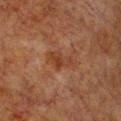– notes — catalogued during a skin exam; not biopsied
– image source — ~15 mm crop, total-body skin-cancer survey
– site — the chest
– patient — female, aged approximately 55
– illumination — cross-polarized illumination
– size — about 4 mm
– automated metrics — an outline eccentricity of about 0.85 (0 = round, 1 = elongated) and two-axis asymmetry of about 0.45; border irregularity of about 5 on a 0–10 scale and a within-lesion color-variation index near 4.5/10